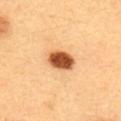Recorded during total-body skin imaging; not selected for excision or biopsy.
The lesion is located on the upper back.
A lesion tile, about 15 mm wide, cut from a 3D total-body photograph.
Automated tile analysis of the lesion measured an area of roughly 7.5 mm² and a shape eccentricity near 0.7. And it measured about 21 CIELAB-L* units darker than the surrounding skin and a normalized border contrast of about 13.5.
Imaged with cross-polarized lighting.
The patient is a female about 30 years old.
Measured at roughly 3.5 mm in maximum diameter.